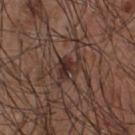Q: Was a biopsy performed?
A: total-body-photography surveillance lesion; no biopsy
Q: What kind of image is this?
A: ~15 mm tile from a whole-body skin photo
Q: Patient demographics?
A: male, about 55 years old
Q: What is the anatomic site?
A: the front of the torso
Q: Lesion size?
A: about 3.5 mm
Q: Automated lesion metrics?
A: an average lesion color of about L≈30 a*≈18 b*≈20 (CIELAB) and about 9 CIELAB-L* units darker than the surrounding skin; lesion-presence confidence of about 95/100